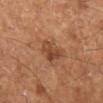<lesion>
<biopsy_status>not biopsied; imaged during a skin examination</biopsy_status>
<lesion_size>
  <long_diameter_mm_approx>3.0</long_diameter_mm_approx>
</lesion_size>
<image>
  <source>total-body photography crop</source>
  <field_of_view_mm>15</field_of_view_mm>
</image>
<site>leg</site>
<patient>
  <sex>male</sex>
  <age_approx>65</age_approx>
</patient>
<automated_metrics>
  <cielab_L>45</cielab_L>
  <cielab_a>23</cielab_a>
  <cielab_b>32</cielab_b>
  <vs_skin_darker_L>9.0</vs_skin_darker_L>
  <peripheral_color_asymmetry>1.5</peripheral_color_asymmetry>
  <nevus_likeness_0_100>60</nevus_likeness_0_100>
</automated_metrics>
<lighting>cross-polarized</lighting>
</lesion>